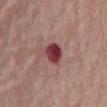Assessment: Recorded during total-body skin imaging; not selected for excision or biopsy.